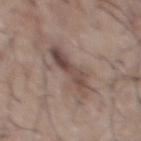| feature | finding |
|---|---|
| biopsy status | catalogued during a skin exam; not biopsied |
| lighting | white-light |
| acquisition | total-body-photography crop, ~15 mm field of view |
| size | about 5 mm |
| body site | the chest |
| image-analysis metrics | a footprint of about 10 mm², an outline eccentricity of about 0.9 (0 = round, 1 = elongated), and a shape-asymmetry score of about 0.3 (0 = symmetric); a lesion color around L≈47 a*≈16 b*≈21 in CIELAB, about 10 CIELAB-L* units darker than the surrounding skin, and a lesion-to-skin contrast of about 8 (normalized; higher = more distinct); internal color variation of about 7.5 on a 0–10 scale and peripheral color asymmetry of about 3; a detector confidence of about 100 out of 100 that the crop contains a lesion |
| subject | male, aged around 75 |